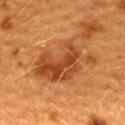- follow-up: no biopsy performed (imaged during a skin exam)
- site: the upper back
- subject: female, approximately 40 years of age
- image source: total-body-photography crop, ~15 mm field of view